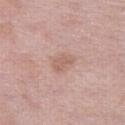Captured during whole-body skin photography for melanoma surveillance; the lesion was not biopsied. A female subject, about 40 years old. A 15 mm close-up tile from a total-body photography series done for melanoma screening. On the leg.